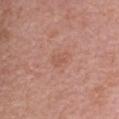Q: Is there a histopathology result?
A: total-body-photography surveillance lesion; no biopsy
Q: What are the patient's age and sex?
A: female, about 65 years old
Q: How was this image acquired?
A: ~15 mm tile from a whole-body skin photo
Q: How was the tile lit?
A: white-light illumination
Q: Lesion size?
A: about 3 mm
Q: What is the anatomic site?
A: the upper back